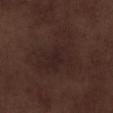No biopsy was performed on this lesion — it was imaged during a full skin examination and was not determined to be concerning. A close-up tile cropped from a whole-body skin photograph, about 15 mm across. Measured at roughly 7 mm in maximum diameter. A male subject about 70 years old. Automated tile analysis of the lesion measured an eccentricity of roughly 0.65 and a symmetry-axis asymmetry near 0.45. And it measured a border-irregularity index near 6.5/10, internal color variation of about 2 on a 0–10 scale, and radial color variation of about 0.5. And it measured an automated nevus-likeness rating near 0 out of 100 and a lesion-detection confidence of about 100/100. The lesion is on the right lower leg. Imaged with white-light lighting.